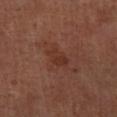{
  "biopsy_status": "not biopsied; imaged during a skin examination",
  "image": {
    "source": "total-body photography crop",
    "field_of_view_mm": 15
  },
  "lesion_size": {
    "long_diameter_mm_approx": 3.0
  },
  "site": "right lower leg",
  "automated_metrics": {
    "border_irregularity_0_10": 3.0,
    "color_variation_0_10": 2.5,
    "peripheral_color_asymmetry": 1.0,
    "nevus_likeness_0_100": 0,
    "lesion_detection_confidence_0_100": 100
  },
  "lighting": "cross-polarized",
  "patient": {
    "sex": "male",
    "age_approx": 65
  }
}